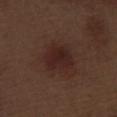Recorded during total-body skin imaging; not selected for excision or biopsy. The lesion is located on the left thigh. A male subject, aged approximately 70. Imaged with white-light lighting. Approximately 4 mm at its widest. The lesion-visualizer software estimated peripheral color asymmetry of about 1. A 15 mm close-up tile from a total-body photography series done for melanoma screening.